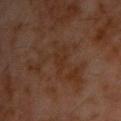Notes:
- follow-up — total-body-photography surveillance lesion; no biopsy
- body site — the chest
- tile lighting — cross-polarized
- patient — male, aged approximately 60
- automated lesion analysis — an area of roughly 2.5 mm²; a classifier nevus-likeness of about 0/100 and a lesion-detection confidence of about 100/100
- image — total-body-photography crop, ~15 mm field of view